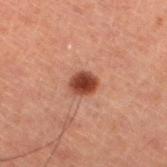Imaged during a routine full-body skin examination; the lesion was not biopsied and no histopathology is available.
A 15 mm close-up extracted from a 3D total-body photography capture.
Captured under cross-polarized illumination.
The lesion's longest dimension is about 2.5 mm.
A male subject, aged approximately 60.
From the left lower leg.